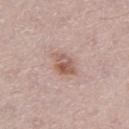Findings:
• follow-up — no biopsy performed (imaged during a skin exam)
• imaging modality — ~15 mm tile from a whole-body skin photo
• subject — male, aged approximately 65
• lesion size — ≈3.5 mm
• TBP lesion metrics — an area of roughly 6 mm², an outline eccentricity of about 0.7 (0 = round, 1 = elongated), and a shape-asymmetry score of about 0.2 (0 = symmetric); an average lesion color of about L≈57 a*≈20 b*≈26 (CIELAB), a lesion–skin lightness drop of about 10, and a normalized border contrast of about 7.5; a border-irregularity index near 2/10, a within-lesion color-variation index near 6.5/10, and a peripheral color-asymmetry measure near 2; an automated nevus-likeness rating near 80 out of 100
• anatomic site — the left thigh